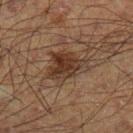Assessment: No biopsy was performed on this lesion — it was imaged during a full skin examination and was not determined to be concerning. Acquisition and patient details: A region of skin cropped from a whole-body photographic capture, roughly 15 mm wide. An algorithmic analysis of the crop reported a footprint of about 14 mm² and two-axis asymmetry of about 0.35. The software also gave a mean CIELAB color near L≈27 a*≈13 b*≈22, a lesion–skin lightness drop of about 8, and a lesion-to-skin contrast of about 8.5 (normalized; higher = more distinct). And it measured a border-irregularity index near 4.5/10. And it measured a classifier nevus-likeness of about 70/100 and a detector confidence of about 100 out of 100 that the crop contains a lesion. Approximately 5.5 mm at its widest. Imaged with cross-polarized lighting. The lesion is located on the left lower leg. A male subject, aged 48–52.